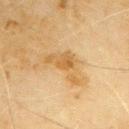No biopsy was performed on this lesion — it was imaged during a full skin examination and was not determined to be concerning.
Located on the arm.
The patient is a male aged approximately 70.
A roughly 15 mm field-of-view crop from a total-body skin photograph.